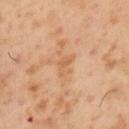Recorded during total-body skin imaging; not selected for excision or biopsy. A lesion tile, about 15 mm wide, cut from a 3D total-body photograph. On the left upper arm. The subject is a male approximately 55 years of age.